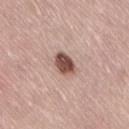This lesion was catalogued during total-body skin photography and was not selected for biopsy. The lesion's longest dimension is about 3.5 mm. The tile uses white-light illumination. Located on the lower back. Automated image analysis of the tile measured a footprint of about 6 mm² and a shape eccentricity near 0.75. The software also gave border irregularity of about 2 on a 0–10 scale, a within-lesion color-variation index near 4.5/10, and peripheral color asymmetry of about 1.5. A female subject roughly 50 years of age. A 15 mm crop from a total-body photograph taken for skin-cancer surveillance.